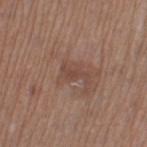The lesion was tiled from a total-body skin photograph and was not biopsied.
Captured under white-light illumination.
The lesion is located on the leg.
Cropped from a whole-body photographic skin survey; the tile spans about 15 mm.
The lesion-visualizer software estimated an automated nevus-likeness rating near 0 out of 100 and a detector confidence of about 100 out of 100 that the crop contains a lesion.
The lesion's longest dimension is about 3 mm.
A female subject aged 53 to 57.